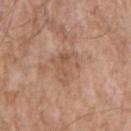image source=~15 mm crop, total-body skin-cancer survey | size=about 4.5 mm | subject=male, about 60 years old | location=the left upper arm.